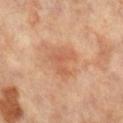Captured during whole-body skin photography for melanoma surveillance; the lesion was not biopsied. From the left leg. Cropped from a whole-body photographic skin survey; the tile spans about 15 mm. The lesion-visualizer software estimated an area of roughly 5.5 mm² and two-axis asymmetry of about 0.55. It also reported a border-irregularity index near 6.5/10, internal color variation of about 2 on a 0–10 scale, and peripheral color asymmetry of about 0.5. It also reported a detector confidence of about 100 out of 100 that the crop contains a lesion. The subject is a female roughly 65 years of age. Approximately 3.5 mm at its widest.